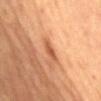Impression:
Imaged during a routine full-body skin examination; the lesion was not biopsied and no histopathology is available.
Clinical summary:
A roughly 15 mm field-of-view crop from a total-body skin photograph. The lesion's longest dimension is about 3 mm. The tile uses cross-polarized illumination. The lesion is located on the back. The patient is a female aged 58 to 62.